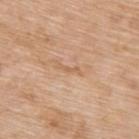| field | value |
|---|---|
| workup | catalogued during a skin exam; not biopsied |